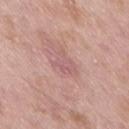Background:
Imaged with white-light lighting. From the right thigh. The recorded lesion diameter is about 3 mm. A lesion tile, about 15 mm wide, cut from a 3D total-body photograph. The subject is a male aged 53 to 57.